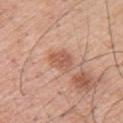The lesion was photographed on a routine skin check and not biopsied; there is no pathology result. This image is a 15 mm lesion crop taken from a total-body photograph. The lesion's longest dimension is about 3 mm. From the upper back. A male patient aged 53–57. Captured under white-light illumination. An algorithmic analysis of the crop reported a shape eccentricity near 0.65 and a symmetry-axis asymmetry near 0.25. The analysis additionally found a mean CIELAB color near L≈57 a*≈24 b*≈31 and a lesion-to-skin contrast of about 6.5 (normalized; higher = more distinct). And it measured border irregularity of about 3 on a 0–10 scale and a color-variation rating of about 2.5/10. It also reported an automated nevus-likeness rating near 5 out of 100.